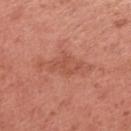Imaged during a routine full-body skin examination; the lesion was not biopsied and no histopathology is available.
Imaged with white-light lighting.
A female patient, aged approximately 50.
The lesion is on the right upper arm.
Cropped from a whole-body photographic skin survey; the tile spans about 15 mm.
Automated tile analysis of the lesion measured about 7 CIELAB-L* units darker than the surrounding skin and a normalized lesion–skin contrast near 5. And it measured a border-irregularity rating of about 5/10 and a within-lesion color-variation index near 2/10. And it measured a nevus-likeness score of about 0/100 and a lesion-detection confidence of about 100/100.
Longest diameter approximately 6.5 mm.